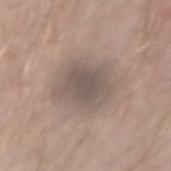Part of a total-body skin-imaging series; this lesion was reviewed on a skin check and was not flagged for biopsy. The patient is a male aged approximately 40. Cropped from a total-body skin-imaging series; the visible field is about 15 mm. This is a white-light tile. Approximately 5 mm at its widest. The lesion is on the right forearm. Automated tile analysis of the lesion measured an area of roughly 16 mm² and a symmetry-axis asymmetry near 0.2. The software also gave a color-variation rating of about 2.5/10 and peripheral color asymmetry of about 0.5. The analysis additionally found lesion-presence confidence of about 65/100.